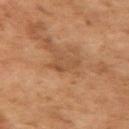site: left upper arm
lesion_size:
  long_diameter_mm_approx: 3.5
lighting: cross-polarized
image:
  source: total-body photography crop
  field_of_view_mm: 15
patient:
  sex: female
  age_approx: 60